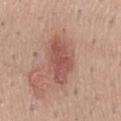biopsy_status: not biopsied; imaged during a skin examination
image:
  source: total-body photography crop
  field_of_view_mm: 15
site: mid back
patient:
  sex: male
  age_approx: 45